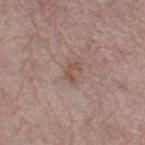notes: no biopsy performed (imaged during a skin exam).